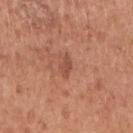Imaged during a routine full-body skin examination; the lesion was not biopsied and no histopathology is available. A roughly 15 mm field-of-view crop from a total-body skin photograph. This is a white-light tile. Located on the right upper arm. The total-body-photography lesion software estimated radial color variation of about 0.5. The recorded lesion diameter is about 2.5 mm. A female patient, aged 48 to 52.